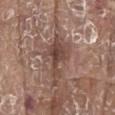| field | value |
|---|---|
| follow-up | no biopsy performed (imaged during a skin exam) |
| acquisition | total-body-photography crop, ~15 mm field of view |
| body site | the chest |
| image-analysis metrics | a border-irregularity index near 7/10, a within-lesion color-variation index near 5/10, and radial color variation of about 1.5; a classifier nevus-likeness of about 0/100 and a detector confidence of about 90 out of 100 that the crop contains a lesion |
| subject | male, aged 78–82 |
| lighting | white-light illumination |